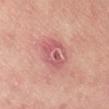tile lighting: white-light
body site: the right thigh
diameter: ~5.5 mm (longest diameter)
automated metrics: a lesion color around L≈59 a*≈29 b*≈23 in CIELAB, about 10 CIELAB-L* units darker than the surrounding skin, and a lesion-to-skin contrast of about 7 (normalized; higher = more distinct); a border-irregularity index near 2/10 and peripheral color asymmetry of about 2; a classifier nevus-likeness of about 0/100 and lesion-presence confidence of about 100/100
imaging modality: 15 mm crop, total-body photography
patient: female, roughly 35 years of age